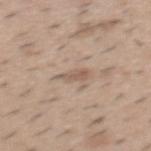The lesion was tiled from a total-body skin photograph and was not biopsied.
About 3 mm across.
The lesion is located on the mid back.
A male subject, in their 40s.
A region of skin cropped from a whole-body photographic capture, roughly 15 mm wide.